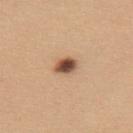| feature | finding |
|---|---|
| notes | imaged on a skin check; not biopsied |
| image source | 15 mm crop, total-body photography |
| patient | female, aged approximately 40 |
| TBP lesion metrics | a mean CIELAB color near L≈51 a*≈20 b*≈30, about 19 CIELAB-L* units darker than the surrounding skin, and a lesion-to-skin contrast of about 12.5 (normalized; higher = more distinct); a border-irregularity rating of about 1.5/10 and a color-variation rating of about 5/10; an automated nevus-likeness rating near 100 out of 100 |
| anatomic site | the upper back |
| lighting | white-light |